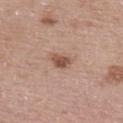biopsy status: catalogued during a skin exam; not biopsied | location: the upper back | illumination: white-light illumination | image source: 15 mm crop, total-body photography | subject: female, about 65 years old | automated metrics: a lesion area of about 3.5 mm², a shape eccentricity near 0.8, and a symmetry-axis asymmetry near 0.25; an average lesion color of about L≈51 a*≈21 b*≈27 (CIELAB), a lesion–skin lightness drop of about 12, and a normalized lesion–skin contrast near 8.5; border irregularity of about 2.5 on a 0–10 scale and a within-lesion color-variation index near 2.5/10; an automated nevus-likeness rating near 80 out of 100 and a lesion-detection confidence of about 100/100.